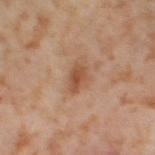Captured during whole-body skin photography for melanoma surveillance; the lesion was not biopsied. Automated image analysis of the tile measured about 10 CIELAB-L* units darker than the surrounding skin and a normalized lesion–skin contrast near 7.5. The software also gave a nevus-likeness score of about 5/100 and lesion-presence confidence of about 100/100. Captured under cross-polarized illumination. The recorded lesion diameter is about 3 mm. Cropped from a total-body skin-imaging series; the visible field is about 15 mm. The subject is a female aged approximately 55. The lesion is on the right thigh.